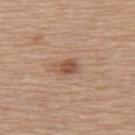<tbp_lesion>
<site>upper back</site>
<lighting>white-light</lighting>
<automated_metrics>
  <border_irregularity_0_10>2.5</border_irregularity_0_10>
  <peripheral_color_asymmetry>1.5</peripheral_color_asymmetry>
</automated_metrics>
<patient>
  <sex>female</sex>
  <age_approx>65</age_approx>
</patient>
<image>
  <source>total-body photography crop</source>
  <field_of_view_mm>15</field_of_view_mm>
</image>
<lesion_size>
  <long_diameter_mm_approx>3.0</long_diameter_mm_approx>
</lesion_size>
</tbp_lesion>